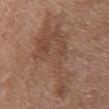Captured during whole-body skin photography for melanoma surveillance; the lesion was not biopsied.
A 15 mm close-up tile from a total-body photography series done for melanoma screening.
The lesion is on the upper back.
A female patient, aged 68–72.